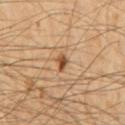This lesion was catalogued during total-body skin photography and was not selected for biopsy. About 2 mm across. A male patient, aged approximately 55. An algorithmic analysis of the crop reported a footprint of about 2.5 mm², a shape eccentricity near 0.65, and a symmetry-axis asymmetry near 0.3. The software also gave an average lesion color of about L≈48 a*≈21 b*≈34 (CIELAB). The software also gave a border-irregularity index near 2.5/10, a within-lesion color-variation index near 4/10, and a peripheral color-asymmetry measure near 1. It also reported a classifier nevus-likeness of about 95/100 and a lesion-detection confidence of about 100/100. Cropped from a whole-body photographic skin survey; the tile spans about 15 mm. From the chest.